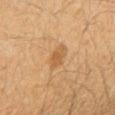Captured during whole-body skin photography for melanoma surveillance; the lesion was not biopsied. A lesion tile, about 15 mm wide, cut from a 3D total-body photograph. The recorded lesion diameter is about 3 mm. The tile uses cross-polarized illumination. The lesion is on the right forearm. A female subject aged 38 to 42.